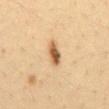No biopsy was performed on this lesion — it was imaged during a full skin examination and was not determined to be concerning. A region of skin cropped from a whole-body photographic capture, roughly 15 mm wide. About 3.5 mm across. The lesion is located on the front of the torso. Automated tile analysis of the lesion measured an area of roughly 5 mm², an eccentricity of roughly 0.85, and two-axis asymmetry of about 0.2. The analysis additionally found a color-variation rating of about 5/10 and peripheral color asymmetry of about 1.5. It also reported an automated nevus-likeness rating near 100 out of 100 and a detector confidence of about 100 out of 100 that the crop contains a lesion. A male subject in their mid-30s. This is a cross-polarized tile.